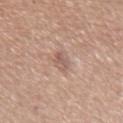Captured during whole-body skin photography for melanoma surveillance; the lesion was not biopsied. From the right forearm. This image is a 15 mm lesion crop taken from a total-body photograph. This is a white-light tile. The patient is a female aged 53 to 57.